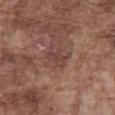A male patient in their mid- to late 70s. Located on the front of the torso. Longest diameter approximately 3 mm. A roughly 15 mm field-of-view crop from a total-body skin photograph.